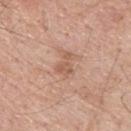Impression:
The lesion was tiled from a total-body skin photograph and was not biopsied.
Context:
On the upper back. The recorded lesion diameter is about 3 mm. A male subject about 60 years old. An algorithmic analysis of the crop reported an outline eccentricity of about 0.8 (0 = round, 1 = elongated) and two-axis asymmetry of about 0.55. The analysis additionally found border irregularity of about 6.5 on a 0–10 scale, internal color variation of about 1.5 on a 0–10 scale, and peripheral color asymmetry of about 0.5. The software also gave a nevus-likeness score of about 0/100 and lesion-presence confidence of about 100/100. Captured under white-light illumination. Cropped from a total-body skin-imaging series; the visible field is about 15 mm.